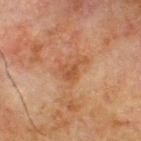Clinical impression: The lesion was photographed on a routine skin check and not biopsied; there is no pathology result. Clinical summary: The total-body-photography lesion software estimated a border-irregularity rating of about 4.5/10 and a color-variation rating of about 3.5/10. And it measured a nevus-likeness score of about 0/100 and a lesion-detection confidence of about 100/100. This image is a 15 mm lesion crop taken from a total-body photograph. The tile uses cross-polarized illumination. Located on the back. A male patient, about 70 years old. The recorded lesion diameter is about 2.5 mm.